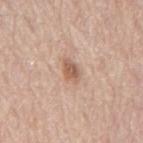Assessment: Imaged during a routine full-body skin examination; the lesion was not biopsied and no histopathology is available. Image and clinical context: The tile uses white-light illumination. A male patient, about 80 years old. The lesion's longest dimension is about 3 mm. An algorithmic analysis of the crop reported an area of roughly 4.5 mm², a shape eccentricity near 0.8, and a symmetry-axis asymmetry near 0.2. The analysis additionally found a nevus-likeness score of about 90/100. A 15 mm close-up tile from a total-body photography series done for melanoma screening. Located on the back.